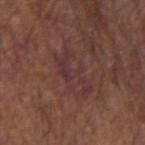Assessment:
Imaged during a routine full-body skin examination; the lesion was not biopsied and no histopathology is available.
Acquisition and patient details:
A 15 mm close-up extracted from a 3D total-body photography capture. Imaged with white-light lighting. An algorithmic analysis of the crop reported a footprint of about 14 mm², an outline eccentricity of about 0.85 (0 = round, 1 = elongated), and a symmetry-axis asymmetry near 0.3. The analysis additionally found a mean CIELAB color near L≈35 a*≈20 b*≈19, roughly 4 lightness units darker than nearby skin, and a lesion-to-skin contrast of about 6 (normalized; higher = more distinct). The recorded lesion diameter is about 6 mm. The lesion is on the left forearm. A male subject roughly 65 years of age.